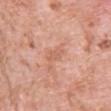follow-up = no biopsy performed (imaged during a skin exam) | diameter = ~3 mm (longest diameter) | automated metrics = a symmetry-axis asymmetry near 0.4 | body site = the chest | lighting = white-light | imaging modality = ~15 mm tile from a whole-body skin photo | patient = male, aged around 50.